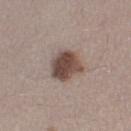| feature | finding |
|---|---|
| workup | catalogued during a skin exam; not biopsied |
| body site | the left thigh |
| automated lesion analysis | a lesion color around L≈46 a*≈16 b*≈22 in CIELAB and a lesion–skin lightness drop of about 15; a border-irregularity rating of about 2/10, internal color variation of about 4.5 on a 0–10 scale, and a peripheral color-asymmetry measure near 1.5; an automated nevus-likeness rating near 95 out of 100 |
| subject | female, aged 38 to 42 |
| image source | ~15 mm crop, total-body skin-cancer survey |
| illumination | white-light |
| lesion size | ~3.5 mm (longest diameter) |